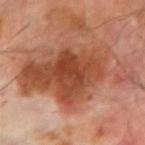{
  "biopsy_status": "not biopsied; imaged during a skin examination",
  "patient": {
    "sex": "male",
    "age_approx": 70
  },
  "image": {
    "source": "total-body photography crop",
    "field_of_view_mm": 15
  },
  "automated_metrics": {
    "area_mm2_approx": 55.0,
    "eccentricity": 0.9,
    "border_irregularity_0_10": 6.0,
    "nevus_likeness_0_100": 10,
    "lesion_detection_confidence_0_100": 100
  },
  "lighting": "cross-polarized",
  "site": "arm"
}